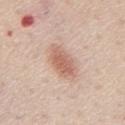No biopsy was performed on this lesion — it was imaged during a full skin examination and was not determined to be concerning.
The lesion's longest dimension is about 4.5 mm.
A male patient aged around 60.
The lesion is located on the back.
This image is a 15 mm lesion crop taken from a total-body photograph.